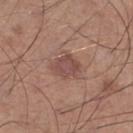Assessment: Captured during whole-body skin photography for melanoma surveillance; the lesion was not biopsied. Context: An algorithmic analysis of the crop reported a lesion area of about 6.5 mm², an outline eccentricity of about 0.7 (0 = round, 1 = elongated), and two-axis asymmetry of about 0.2. And it measured border irregularity of about 2 on a 0–10 scale, a color-variation rating of about 2/10, and a peripheral color-asymmetry measure near 0.5. The software also gave an automated nevus-likeness rating near 15 out of 100 and lesion-presence confidence of about 100/100. A male patient, aged 58 to 62. About 3.5 mm across. The lesion is located on the left lower leg. A roughly 15 mm field-of-view crop from a total-body skin photograph. Imaged with white-light lighting.